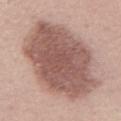The lesion was photographed on a routine skin check and not biopsied; there is no pathology result.